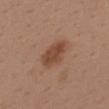biopsy_status: not biopsied; imaged during a skin examination
patient:
  sex: male
  age_approx: 40
image:
  source: total-body photography crop
  field_of_view_mm: 15
site: upper back